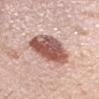<tbp_lesion>
  <site>head or neck</site>
  <patient>
    <sex>male</sex>
    <age_approx>35</age_approx>
  </patient>
  <image>
    <source>total-body photography crop</source>
    <field_of_view_mm>15</field_of_view_mm>
  </image>
  <lesion_size>
    <long_diameter_mm_approx>5.5</long_diameter_mm_approx>
  </lesion_size>
</tbp_lesion>